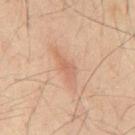<tbp_lesion>
<biopsy_status>not biopsied; imaged during a skin examination</biopsy_status>
<lighting>cross-polarized</lighting>
<site>mid back</site>
<automated_metrics>
  <area_mm2_approx>4.0</area_mm2_approx>
  <cielab_L>55</cielab_L>
  <cielab_a>20</cielab_a>
  <cielab_b>29</cielab_b>
  <vs_skin_darker_L>7.0</vs_skin_darker_L>
</automated_metrics>
<patient>
  <sex>male</sex>
  <age_approx>50</age_approx>
</patient>
<lesion_size>
  <long_diameter_mm_approx>3.5</long_diameter_mm_approx>
</lesion_size>
<image>
  <source>total-body photography crop</source>
  <field_of_view_mm>15</field_of_view_mm>
</image>
</tbp_lesion>